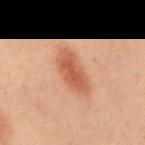The lesion was tiled from a total-body skin photograph and was not biopsied. This is a cross-polarized tile. Located on the back. A 15 mm crop from a total-body photograph taken for skin-cancer surveillance. Approximately 5.5 mm at its widest. A male subject, about 60 years old. Automated image analysis of the tile measured an average lesion color of about L≈46 a*≈23 b*≈29 (CIELAB), roughly 10 lightness units darker than nearby skin, and a lesion-to-skin contrast of about 7.5 (normalized; higher = more distinct). The software also gave a border-irregularity rating of about 2.5/10, a color-variation rating of about 3/10, and a peripheral color-asymmetry measure near 1. And it measured a classifier nevus-likeness of about 95/100 and lesion-presence confidence of about 100/100.